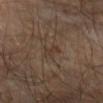The lesion was photographed on a routine skin check and not biopsied; there is no pathology result.